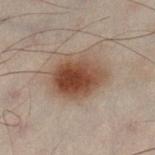Clinical impression:
Captured during whole-body skin photography for melanoma surveillance; the lesion was not biopsied.
Acquisition and patient details:
The tile uses cross-polarized illumination. The patient is a male about 50 years old. A 15 mm close-up tile from a total-body photography series done for melanoma screening. About 5.5 mm across. Automated tile analysis of the lesion measured a mean CIELAB color near L≈37 a*≈16 b*≈24, a lesion–skin lightness drop of about 12, and a normalized border contrast of about 11. And it measured a border-irregularity index near 1.5/10 and internal color variation of about 6 on a 0–10 scale. The lesion is on the left lower leg.